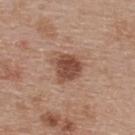Context:
A female patient, aged 38–42. The lesion is located on the back. A lesion tile, about 15 mm wide, cut from a 3D total-body photograph. The tile uses white-light illumination.